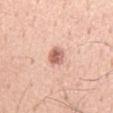workup — no biopsy performed (imaged during a skin exam) | body site — the chest | size — about 2.5 mm | tile lighting — white-light | image — 15 mm crop, total-body photography | subject — male, aged approximately 55 | TBP lesion metrics — a footprint of about 4.5 mm² and an eccentricity of roughly 0.75; a lesion–skin lightness drop of about 15 and a lesion-to-skin contrast of about 8.5 (normalized; higher = more distinct); a border-irregularity index near 1.5/10, internal color variation of about 4.5 on a 0–10 scale, and a peripheral color-asymmetry measure near 1.5.